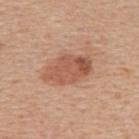No biopsy was performed on this lesion — it was imaged during a full skin examination and was not determined to be concerning.
Imaged with white-light lighting.
A 15 mm close-up tile from a total-body photography series done for melanoma screening.
A female patient, aged 48 to 52.
Located on the upper back.
The recorded lesion diameter is about 5.5 mm.